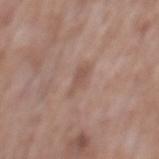Assessment: Captured during whole-body skin photography for melanoma surveillance; the lesion was not biopsied.